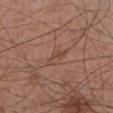This lesion was catalogued during total-body skin photography and was not selected for biopsy.
Cropped from a whole-body photographic skin survey; the tile spans about 15 mm.
Captured under white-light illumination.
Located on the right forearm.
Automated image analysis of the tile measured a footprint of about 3.5 mm² and a symmetry-axis asymmetry near 0.45. The software also gave an average lesion color of about L≈46 a*≈20 b*≈27 (CIELAB), roughly 6 lightness units darker than nearby skin, and a normalized lesion–skin contrast near 5. The analysis additionally found a border-irregularity index near 5/10 and peripheral color asymmetry of about 0. And it measured an automated nevus-likeness rating near 0 out of 100 and lesion-presence confidence of about 60/100.
Approximately 3.5 mm at its widest.
A male patient, in their mid-50s.